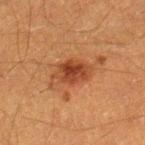Case summary:
– biopsy status · no biopsy performed (imaged during a skin exam)
– lesion diameter · ~4.5 mm (longest diameter)
– anatomic site · the right lower leg
– imaging modality · 15 mm crop, total-body photography
– subject · male, aged approximately 40